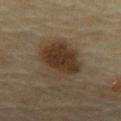Captured during whole-body skin photography for melanoma surveillance; the lesion was not biopsied.